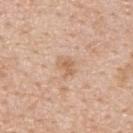The lesion is located on the back. A male subject roughly 50 years of age. Imaged with white-light lighting. A 15 mm close-up extracted from a 3D total-body photography capture.